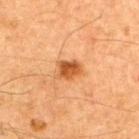Q: Was a biopsy performed?
A: imaged on a skin check; not biopsied
Q: What did automated image analysis measure?
A: an area of roughly 5.5 mm², an eccentricity of roughly 0.55, and a symmetry-axis asymmetry near 0.25
Q: Patient demographics?
A: male, aged around 65
Q: Lesion location?
A: the upper back
Q: Illumination type?
A: cross-polarized illumination
Q: What is the imaging modality?
A: total-body-photography crop, ~15 mm field of view
Q: What is the lesion's diameter?
A: about 3 mm